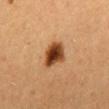Q: Is there a histopathology result?
A: imaged on a skin check; not biopsied
Q: How was this image acquired?
A: 15 mm crop, total-body photography
Q: Where on the body is the lesion?
A: the mid back
Q: What is the lesion's diameter?
A: about 3.5 mm
Q: Patient demographics?
A: female, about 40 years old
Q: Illumination type?
A: cross-polarized illumination